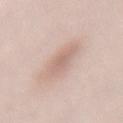<case>
<biopsy_status>not biopsied; imaged during a skin examination</biopsy_status>
<automated_metrics>
  <cielab_L>66</cielab_L>
  <cielab_a>17</cielab_a>
  <cielab_b>25</cielab_b>
  <vs_skin_darker_L>9.0</vs_skin_darker_L>
  <vs_skin_contrast_norm>5.5</vs_skin_contrast_norm>
  <border_irregularity_0_10>2.0</border_irregularity_0_10>
  <color_variation_0_10>2.5</color_variation_0_10>
</automated_metrics>
<image>
  <source>total-body photography crop</source>
  <field_of_view_mm>15</field_of_view_mm>
</image>
<site>lower back</site>
<lighting>white-light</lighting>
<patient>
  <sex>female</sex>
  <age_approx>55</age_approx>
</patient>
</case>